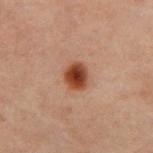<tbp_lesion>
<biopsy_status>not biopsied; imaged during a skin examination</biopsy_status>
<lesion_size>
  <long_diameter_mm_approx>3.0</long_diameter_mm_approx>
</lesion_size>
<site>front of the torso</site>
<image>
  <source>total-body photography crop</source>
  <field_of_view_mm>15</field_of_view_mm>
</image>
<patient>
  <sex>male</sex>
  <age_approx>60</age_approx>
</patient>
<lighting>cross-polarized</lighting>
<automated_metrics>
  <area_mm2_approx>7.0</area_mm2_approx>
  <eccentricity>0.6</eccentricity>
  <vs_skin_darker_L>13.0</vs_skin_darker_L>
  <color_variation_0_10>5.5</color_variation_0_10>
  <peripheral_color_asymmetry>1.5</peripheral_color_asymmetry>
</automated_metrics>
</tbp_lesion>